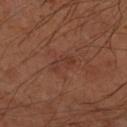The lesion was tiled from a total-body skin photograph and was not biopsied. A roughly 15 mm field-of-view crop from a total-body skin photograph. This is a cross-polarized tile. On the left forearm. The patient is a male in their mid-60s.